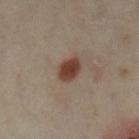{"biopsy_status": "not biopsied; imaged during a skin examination", "automated_metrics": {"cielab_L": 38, "cielab_a": 18, "cielab_b": 24, "vs_skin_darker_L": 13.0}, "lighting": "cross-polarized", "lesion_size": {"long_diameter_mm_approx": 3.0}, "image": {"source": "total-body photography crop", "field_of_view_mm": 15}, "site": "left lower leg", "patient": {"sex": "female", "age_approx": 35}}